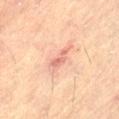Recorded during total-body skin imaging; not selected for excision or biopsy.